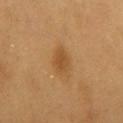• biopsy status — total-body-photography surveillance lesion; no biopsy
• location — the chest
• automated lesion analysis — a lesion color around L≈49 a*≈21 b*≈40 in CIELAB, a lesion–skin lightness drop of about 9, and a normalized lesion–skin contrast near 6.5; a classifier nevus-likeness of about 85/100 and lesion-presence confidence of about 100/100
• lighting — cross-polarized
• subject — male, approximately 60 years of age
• lesion size — ≈3.5 mm
• image — total-body-photography crop, ~15 mm field of view